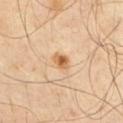Notes:
• biopsy status: total-body-photography surveillance lesion; no biopsy
• subject: male, aged approximately 40
• body site: the front of the torso
• imaging modality: ~15 mm crop, total-body skin-cancer survey
• illumination: cross-polarized
• diameter: ~2.5 mm (longest diameter)
• TBP lesion metrics: a lesion color around L≈63 a*≈21 b*≈40 in CIELAB, roughly 13 lightness units darker than nearby skin, and a normalized border contrast of about 9; an automated nevus-likeness rating near 90 out of 100 and a detector confidence of about 100 out of 100 that the crop contains a lesion